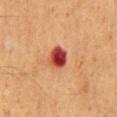Case summary:
* follow-up · catalogued during a skin exam; not biopsied
* image-analysis metrics · a footprint of about 5.5 mm² and an outline eccentricity of about 0.45 (0 = round, 1 = elongated); a mean CIELAB color near L≈38 a*≈32 b*≈28, roughly 17 lightness units darker than nearby skin, and a normalized lesion–skin contrast near 14; a border-irregularity index near 1.5/10; a classifier nevus-likeness of about 0/100 and a lesion-detection confidence of about 100/100
* acquisition · 15 mm crop, total-body photography
* lesion size · about 2.5 mm
* patient · male, approximately 60 years of age
* site · the back
* illumination · cross-polarized illumination